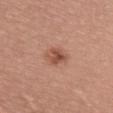biopsy status = total-body-photography surveillance lesion; no biopsy | acquisition = total-body-photography crop, ~15 mm field of view | site = the upper back | subject = female, aged approximately 20.